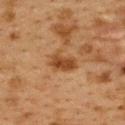Captured during whole-body skin photography for melanoma surveillance; the lesion was not biopsied. Imaged with cross-polarized lighting. A 15 mm close-up extracted from a 3D total-body photography capture. The lesion's longest dimension is about 3.5 mm. The patient is a female in their 40s. An algorithmic analysis of the crop reported an area of roughly 6 mm², a shape eccentricity near 0.8, and a shape-asymmetry score of about 0.35 (0 = symmetric). The software also gave border irregularity of about 3.5 on a 0–10 scale, internal color variation of about 2.5 on a 0–10 scale, and peripheral color asymmetry of about 1. Located on the back.